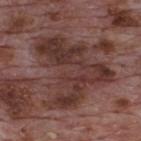The lesion was tiled from a total-body skin photograph and was not biopsied. Cropped from a whole-body photographic skin survey; the tile spans about 15 mm. The lesion is on the upper back. A male patient, aged approximately 70.